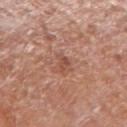workup=total-body-photography surveillance lesion; no biopsy | anatomic site=the right upper arm | image-analysis metrics=a border-irregularity index near 4/10, a within-lesion color-variation index near 1/10, and peripheral color asymmetry of about 0.5; a nevus-likeness score of about 5/100 | image source=~15 mm tile from a whole-body skin photo | size=about 2.5 mm | subject=male, aged 63 to 67 | illumination=white-light.